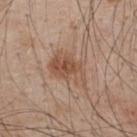workup = imaged on a skin check; not biopsied
anatomic site = the upper back
automated metrics = a footprint of about 12 mm², an eccentricity of roughly 0.8, and a symmetry-axis asymmetry near 0.3; a normalized lesion–skin contrast near 7; a color-variation rating of about 5.5/10 and peripheral color asymmetry of about 2
lighting = white-light illumination
diameter = ~5 mm (longest diameter)
imaging modality = ~15 mm tile from a whole-body skin photo
subject = male, roughly 50 years of age